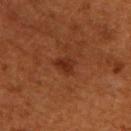{"biopsy_status": "not biopsied; imaged during a skin examination", "site": "upper back", "image": {"source": "total-body photography crop", "field_of_view_mm": 15}, "patient": {"sex": "male", "age_approx": 55}}